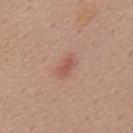Part of a total-body skin-imaging series; this lesion was reviewed on a skin check and was not flagged for biopsy.
Located on the mid back.
Longest diameter approximately 2.5 mm.
A 15 mm crop from a total-body photograph taken for skin-cancer surveillance.
Captured under white-light illumination.
The lesion-visualizer software estimated a footprint of about 3 mm² and a shape-asymmetry score of about 0.35 (0 = symmetric). It also reported a border-irregularity rating of about 3.5/10, a color-variation rating of about 0/10, and peripheral color asymmetry of about 0. The software also gave a nevus-likeness score of about 65/100 and a detector confidence of about 100 out of 100 that the crop contains a lesion.
A male subject in their mid-50s.